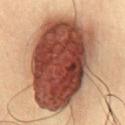Case summary:
* follow-up · no biopsy performed (imaged during a skin exam)
* location · the chest
* subject · male, in their mid- to late 30s
* image source · ~15 mm tile from a whole-body skin photo
* automated lesion analysis · a classifier nevus-likeness of about 100/100
* diameter · about 11.5 mm